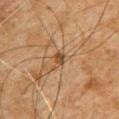– follow-up · total-body-photography surveillance lesion; no biopsy
– TBP lesion metrics · about 9 CIELAB-L* units darker than the surrounding skin and a normalized border contrast of about 7.5
– acquisition · total-body-photography crop, ~15 mm field of view
– site · the chest
– size · ~2.5 mm (longest diameter)
– patient · male, in their 60s